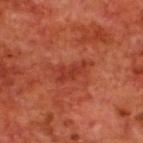<tbp_lesion>
<biopsy_status>not biopsied; imaged during a skin examination</biopsy_status>
<image>
  <source>total-body photography crop</source>
  <field_of_view_mm>15</field_of_view_mm>
</image>
<site>upper back</site>
<automated_metrics>
  <eccentricity>0.9</eccentricity>
  <shape_asymmetry>0.35</shape_asymmetry>
  <vs_skin_darker_L>7.0</vs_skin_darker_L>
  <border_irregularity_0_10>3.5</border_irregularity_0_10>
  <color_variation_0_10>2.0</color_variation_0_10>
  <peripheral_color_asymmetry>0.5</peripheral_color_asymmetry>
  <nevus_likeness_0_100>0</nevus_likeness_0_100>
</automated_metrics>
<patient>
  <sex>male</sex>
  <age_approx>70</age_approx>
</patient>
<lighting>cross-polarized</lighting>
<lesion_size>
  <long_diameter_mm_approx>3.5</long_diameter_mm_approx>
</lesion_size>
</tbp_lesion>